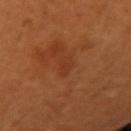| field | value |
|---|---|
| patient | female, aged approximately 50 |
| lesion diameter | about 2.5 mm |
| illumination | cross-polarized illumination |
| image | 15 mm crop, total-body photography |